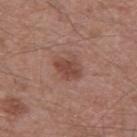<record>
  <biopsy_status>not biopsied; imaged during a skin examination</biopsy_status>
  <site>back</site>
  <lesion_size>
    <long_diameter_mm_approx>3.0</long_diameter_mm_approx>
  </lesion_size>
  <patient>
    <sex>male</sex>
    <age_approx>55</age_approx>
  </patient>
  <image>
    <source>total-body photography crop</source>
    <field_of_view_mm>15</field_of_view_mm>
  </image>
  <lighting>white-light</lighting>
  <automated_metrics>
    <area_mm2_approx>6.0</area_mm2_approx>
    <eccentricity>0.6</eccentricity>
    <shape_asymmetry>0.25</shape_asymmetry>
    <border_irregularity_0_10>2.5</border_irregularity_0_10>
    <color_variation_0_10>2.0</color_variation_0_10>
    <peripheral_color_asymmetry>0.5</peripheral_color_asymmetry>
    <lesion_detection_confidence_0_100>100</lesion_detection_confidence_0_100>
  </automated_metrics>
</record>